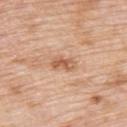workup: imaged on a skin check; not biopsied | body site: the upper back | subject: female, about 70 years old | illumination: white-light illumination | automated lesion analysis: a lesion area of about 4 mm², an outline eccentricity of about 0.9 (0 = round, 1 = elongated), and a symmetry-axis asymmetry near 0.3; roughly 11 lightness units darker than nearby skin; a border-irregularity index near 3/10, a within-lesion color-variation index near 3.5/10, and radial color variation of about 1 | acquisition: ~15 mm tile from a whole-body skin photo.